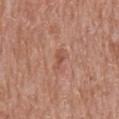Notes:
• subject — male, aged around 65
• lighting — white-light
• lesion size — about 3 mm
• acquisition — total-body-photography crop, ~15 mm field of view
• location — the chest